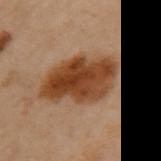Clinical impression: No biopsy was performed on this lesion — it was imaged during a full skin examination and was not determined to be concerning. Clinical summary: From the upper back. Approximately 7 mm at its widest. A female patient, roughly 60 years of age. Automated image analysis of the tile measured an area of roughly 26 mm², a shape eccentricity near 0.8, and a shape-asymmetry score of about 0.2 (0 = symmetric). And it measured a lesion–skin lightness drop of about 13 and a lesion-to-skin contrast of about 11.5 (normalized; higher = more distinct). And it measured a border-irregularity index near 2.5/10 and a peripheral color-asymmetry measure near 2. The analysis additionally found an automated nevus-likeness rating near 100 out of 100 and a detector confidence of about 100 out of 100 that the crop contains a lesion. A 15 mm close-up extracted from a 3D total-body photography capture. Captured under cross-polarized illumination.